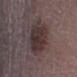No biopsy was performed on this lesion — it was imaged during a full skin examination and was not determined to be concerning. The tile uses white-light illumination. A male patient in their mid-70s. A close-up tile cropped from a whole-body skin photograph, about 15 mm across. Located on the left lower leg. The lesion-visualizer software estimated a footprint of about 18 mm², an eccentricity of roughly 0.65, and a symmetry-axis asymmetry near 0.15. The analysis additionally found border irregularity of about 1.5 on a 0–10 scale, internal color variation of about 5.5 on a 0–10 scale, and a peripheral color-asymmetry measure near 2. And it measured a nevus-likeness score of about 0/100. The lesion's longest dimension is about 5.5 mm.